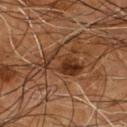Recorded during total-body skin imaging; not selected for excision or biopsy. A lesion tile, about 15 mm wide, cut from a 3D total-body photograph. An algorithmic analysis of the crop reported a footprint of about 11 mm². The analysis additionally found a border-irregularity rating of about 6/10, a color-variation rating of about 8.5/10, and a peripheral color-asymmetry measure near 4. Captured under cross-polarized illumination. The lesion is on the chest. A male patient aged approximately 55. Measured at roughly 5 mm in maximum diameter.